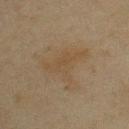No biopsy was performed on this lesion — it was imaged during a full skin examination and was not determined to be concerning. Automated image analysis of the tile measured an outline eccentricity of about 0.7 (0 = round, 1 = elongated) and two-axis asymmetry of about 0.6. The analysis additionally found a detector confidence of about 100 out of 100 that the crop contains a lesion. Located on the chest. A male subject approximately 45 years of age. A 15 mm crop from a total-body photograph taken for skin-cancer surveillance. The tile uses cross-polarized illumination.